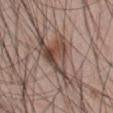* notes · catalogued during a skin exam; not biopsied
* lighting · white-light illumination
* patient · male, aged 43–47
* body site · the abdomen
* acquisition · 15 mm crop, total-body photography
* size · ≈9.5 mm